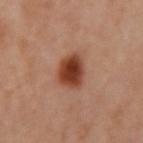Part of a total-body skin-imaging series; this lesion was reviewed on a skin check and was not flagged for biopsy. A region of skin cropped from a whole-body photographic capture, roughly 15 mm wide. A female patient, approximately 50 years of age. The lesion's longest dimension is about 3.5 mm. Located on the arm. This is a cross-polarized tile.